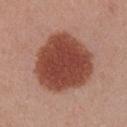Impression:
This lesion was catalogued during total-body skin photography and was not selected for biopsy.